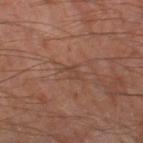Background: The lesion is on the left thigh. The lesion-visualizer software estimated a footprint of about 2 mm² and two-axis asymmetry of about 0.75. The analysis additionally found a mean CIELAB color near L≈39 a*≈18 b*≈25, a lesion–skin lightness drop of about 5, and a normalized lesion–skin contrast near 4.5. Measured at roughly 2.5 mm in maximum diameter. A 15 mm crop from a total-body photograph taken for skin-cancer surveillance. The tile uses cross-polarized illumination. A male subject approximately 55 years of age.